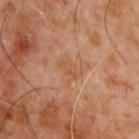Notes:
• workup — no biopsy performed (imaged during a skin exam)
• TBP lesion metrics — border irregularity of about 3.5 on a 0–10 scale and a peripheral color-asymmetry measure near 0.5; an automated nevus-likeness rating near 0 out of 100 and a detector confidence of about 100 out of 100 that the crop contains a lesion
• size — ~3 mm (longest diameter)
• body site — the front of the torso
• image — total-body-photography crop, ~15 mm field of view
• lighting — cross-polarized illumination
• patient — male, in their 60s